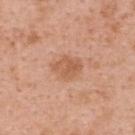Image and clinical context: The recorded lesion diameter is about 3.5 mm. The total-body-photography lesion software estimated a lesion area of about 6.5 mm², an outline eccentricity of about 0.65 (0 = round, 1 = elongated), and two-axis asymmetry of about 0.25. And it measured a mean CIELAB color near L≈58 a*≈24 b*≈34, a lesion–skin lightness drop of about 9, and a lesion-to-skin contrast of about 6 (normalized; higher = more distinct). The analysis additionally found a border-irregularity rating of about 2/10, internal color variation of about 3 on a 0–10 scale, and radial color variation of about 1. Imaged with white-light lighting. On the upper back. The patient is a female about 50 years old. A close-up tile cropped from a whole-body skin photograph, about 15 mm across.